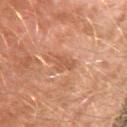Clinical impression: The lesion was photographed on a routine skin check and not biopsied; there is no pathology result. Acquisition and patient details: Longest diameter approximately 3 mm. The lesion is on the left arm. This image is a 15 mm lesion crop taken from a total-body photograph. The tile uses cross-polarized illumination. A male subject, aged approximately 30.